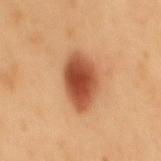biopsy_status: not biopsied; imaged during a skin examination
automated_metrics:
  eccentricity: 0.75
  shape_asymmetry: 0.15
  cielab_L: 51
  cielab_a: 28
  cielab_b: 37
  vs_skin_darker_L: 17.0
  vs_skin_contrast_norm: 11.0
  border_irregularity_0_10: 1.5
  peripheral_color_asymmetry: 1.5
lighting: cross-polarized
patient:
  sex: male
  age_approx: 50
image:
  source: total-body photography crop
  field_of_view_mm: 15
lesion_size:
  long_diameter_mm_approx: 6.0
site: back